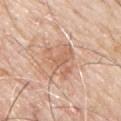Recorded during total-body skin imaging; not selected for excision or biopsy. Automated image analysis of the tile measured a symmetry-axis asymmetry near 0.4. And it measured a lesion color around L≈63 a*≈21 b*≈32 in CIELAB, about 9 CIELAB-L* units darker than the surrounding skin, and a lesion-to-skin contrast of about 5.5 (normalized; higher = more distinct). And it measured a border-irregularity index near 6/10 and a peripheral color-asymmetry measure near 1. The lesion is located on the chest. A lesion tile, about 15 mm wide, cut from a 3D total-body photograph. A male patient, in their mid- to late 60s.